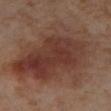Imaged during a routine full-body skin examination; the lesion was not biopsied and no histopathology is available. A female subject, roughly 55 years of age. A 15 mm close-up extracted from a 3D total-body photography capture. The total-body-photography lesion software estimated an area of roughly 70 mm² and an eccentricity of roughly 0.8. The software also gave an average lesion color of about L≈39 a*≈20 b*≈25 (CIELAB) and a normalized border contrast of about 8. It also reported a nevus-likeness score of about 40/100 and a lesion-detection confidence of about 100/100. Longest diameter approximately 13 mm. The lesion is located on the right lower leg.